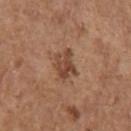Assessment:
This lesion was catalogued during total-body skin photography and was not selected for biopsy.
Acquisition and patient details:
The subject is a female aged 63–67. From the left upper arm. A region of skin cropped from a whole-body photographic capture, roughly 15 mm wide. About 3 mm across. The total-body-photography lesion software estimated an area of roughly 7.5 mm², a shape eccentricity near 0.55, and a shape-asymmetry score of about 0.3 (0 = symmetric). The tile uses white-light illumination.